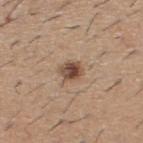Part of a total-body skin-imaging series; this lesion was reviewed on a skin check and was not flagged for biopsy. A male patient in their 60s. Longest diameter approximately 2.5 mm. This image is a 15 mm lesion crop taken from a total-body photograph. Captured under white-light illumination. From the upper back.